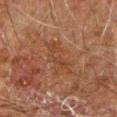| feature | finding |
|---|---|
| workup | total-body-photography surveillance lesion; no biopsy |